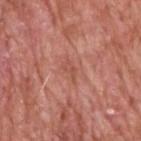Captured during whole-body skin photography for melanoma surveillance; the lesion was not biopsied. The lesion is on the head or neck. The subject is a male aged 58–62. The total-body-photography lesion software estimated an eccentricity of roughly 0.9 and two-axis asymmetry of about 0.55. The software also gave a lesion color around L≈52 a*≈27 b*≈29 in CIELAB and a normalized border contrast of about 5. The analysis additionally found a border-irregularity index near 5.5/10 and a within-lesion color-variation index near 0/10. And it measured an automated nevus-likeness rating near 0 out of 100 and a detector confidence of about 100 out of 100 that the crop contains a lesion. A roughly 15 mm field-of-view crop from a total-body skin photograph. This is a white-light tile.